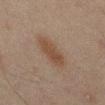  biopsy_status: not biopsied; imaged during a skin examination
  lesion_size:
    long_diameter_mm_approx: 5.0
  lighting: cross-polarized
  patient:
    sex: male
    age_approx: 45
  image:
    source: total-body photography crop
    field_of_view_mm: 15
  site: abdomen